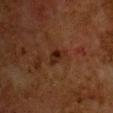Imaged during a routine full-body skin examination; the lesion was not biopsied and no histopathology is available.
A female subject aged approximately 50.
The tile uses cross-polarized illumination.
A region of skin cropped from a whole-body photographic capture, roughly 15 mm wide.
Located on the chest.
Approximately 2.5 mm at its widest.
An algorithmic analysis of the crop reported a footprint of about 3.5 mm², an outline eccentricity of about 0.75 (0 = round, 1 = elongated), and two-axis asymmetry of about 0.4.